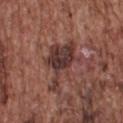This lesion was catalogued during total-body skin photography and was not selected for biopsy.
A 15 mm close-up extracted from a 3D total-body photography capture.
Measured at roughly 6 mm in maximum diameter.
From the back.
A male subject approximately 75 years of age.